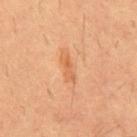Captured during whole-body skin photography for melanoma surveillance; the lesion was not biopsied. A lesion tile, about 15 mm wide, cut from a 3D total-body photograph. A male patient, aged 53–57. Located on the back. About 3 mm across.